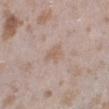{"biopsy_status": "not biopsied; imaged during a skin examination", "automated_metrics": {"color_variation_0_10": 1.5, "peripheral_color_asymmetry": 0.5}, "image": {"source": "total-body photography crop", "field_of_view_mm": 15}, "patient": {"sex": "female", "age_approx": 25}, "site": "right lower leg"}